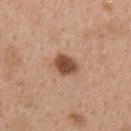Context:
The tile uses white-light illumination. A close-up tile cropped from a whole-body skin photograph, about 15 mm across. About 3 mm across. A female patient aged 38–42. The lesion is located on the right upper arm.